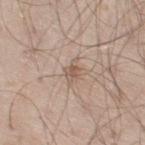This lesion was catalogued during total-body skin photography and was not selected for biopsy.
A 15 mm close-up tile from a total-body photography series done for melanoma screening.
The lesion is on the leg.
A male patient about 60 years old.
The total-body-photography lesion software estimated a footprint of about 3.5 mm², a shape eccentricity near 0.75, and a shape-asymmetry score of about 0.3 (0 = symmetric). The software also gave a border-irregularity index near 3/10, internal color variation of about 3 on a 0–10 scale, and peripheral color asymmetry of about 1.